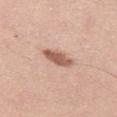biopsy status: imaged on a skin check; not biopsied
lesion diameter: about 4 mm
TBP lesion metrics: border irregularity of about 2.5 on a 0–10 scale, internal color variation of about 3.5 on a 0–10 scale, and radial color variation of about 1; a classifier nevus-likeness of about 85/100 and a detector confidence of about 100 out of 100 that the crop contains a lesion
anatomic site: the left thigh
patient: female, roughly 40 years of age
acquisition: 15 mm crop, total-body photography